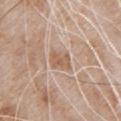{
  "biopsy_status": "not biopsied; imaged during a skin examination",
  "site": "chest",
  "image": {
    "source": "total-body photography crop",
    "field_of_view_mm": 15
  },
  "patient": {
    "sex": "male",
    "age_approx": 80
  }
}